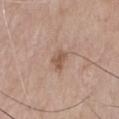Notes:
– biopsy status: total-body-photography surveillance lesion; no biopsy
– anatomic site: the front of the torso
– imaging modality: 15 mm crop, total-body photography
– patient: male, in their mid-70s